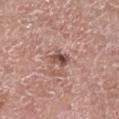Impression: No biopsy was performed on this lesion — it was imaged during a full skin examination and was not determined to be concerning. Image and clinical context: Located on the leg. The lesion's longest dimension is about 2.5 mm. The tile uses white-light illumination. A 15 mm close-up extracted from a 3D total-body photography capture. A male subject aged approximately 75. Automated image analysis of the tile measured a shape eccentricity near 0.75 and a shape-asymmetry score of about 0.25 (0 = symmetric). The analysis additionally found a lesion color around L≈50 a*≈21 b*≈24 in CIELAB, a lesion–skin lightness drop of about 13, and a lesion-to-skin contrast of about 9 (normalized; higher = more distinct). And it measured a classifier nevus-likeness of about 5/100 and lesion-presence confidence of about 100/100.